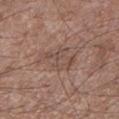The lesion was photographed on a routine skin check and not biopsied; there is no pathology result. Measured at roughly 4 mm in maximum diameter. On the left lower leg. Captured under white-light illumination. A male subject about 55 years old. Cropped from a total-body skin-imaging series; the visible field is about 15 mm.